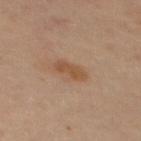No biopsy was performed on this lesion — it was imaged during a full skin examination and was not determined to be concerning. A 15 mm crop from a total-body photograph taken for skin-cancer surveillance. The patient is a female roughly 45 years of age. Automated image analysis of the tile measured an area of roughly 5 mm² and an eccentricity of roughly 0.9. The lesion is located on the mid back.